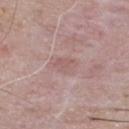| key | value |
|---|---|
| follow-up | no biopsy performed (imaged during a skin exam) |